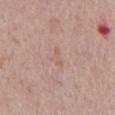Case summary:
– subject · male, roughly 70 years of age
– automated metrics · an area of roughly 2.5 mm², an eccentricity of roughly 0.95, and a symmetry-axis asymmetry near 0.4; a lesion color around L≈60 a*≈19 b*≈27 in CIELAB and a normalized border contrast of about 4.5; a nevus-likeness score of about 0/100 and lesion-presence confidence of about 100/100
– lighting · white-light illumination
– imaging modality · ~15 mm tile from a whole-body skin photo
– size · about 2.5 mm
– body site · the front of the torso